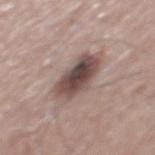| field | value |
|---|---|
| notes | total-body-photography surveillance lesion; no biopsy |
| automated metrics | an outline eccentricity of about 0.8 (0 = round, 1 = elongated) and a symmetry-axis asymmetry near 0.15; about 15 CIELAB-L* units darker than the surrounding skin and a normalized lesion–skin contrast near 11; an automated nevus-likeness rating near 95 out of 100 and a detector confidence of about 100 out of 100 that the crop contains a lesion |
| anatomic site | the mid back |
| diameter | ~5.5 mm (longest diameter) |
| image | ~15 mm crop, total-body skin-cancer survey |
| tile lighting | white-light illumination |
| subject | male, aged 73 to 77 |